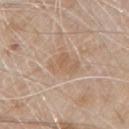<lesion>
  <biopsy_status>not biopsied; imaged during a skin examination</biopsy_status>
  <lighting>white-light</lighting>
  <patient>
    <sex>male</sex>
    <age_approx>80</age_approx>
  </patient>
  <site>front of the torso</site>
  <image>
    <source>total-body photography crop</source>
    <field_of_view_mm>15</field_of_view_mm>
  </image>
  <lesion_size>
    <long_diameter_mm_approx>3.5</long_diameter_mm_approx>
  </lesion_size>
</lesion>